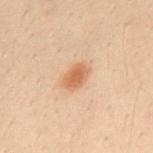The lesion was photographed on a routine skin check and not biopsied; there is no pathology result. The total-body-photography lesion software estimated a mean CIELAB color near L≈51 a*≈19 b*≈31, a lesion–skin lightness drop of about 9, and a normalized border contrast of about 7.5. The software also gave border irregularity of about 1.5 on a 0–10 scale, a color-variation rating of about 2.5/10, and peripheral color asymmetry of about 0.5. And it measured a detector confidence of about 100 out of 100 that the crop contains a lesion. A male patient, aged 28 to 32. Located on the back. A lesion tile, about 15 mm wide, cut from a 3D total-body photograph. Imaged with cross-polarized lighting. The lesion's longest dimension is about 3 mm.